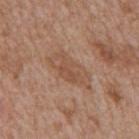Case summary:
– follow-up — total-body-photography surveillance lesion; no biopsy
– lesion diameter — ≈6 mm
– image-analysis metrics — a footprint of about 12 mm², an outline eccentricity of about 0.9 (0 = round, 1 = elongated), and a shape-asymmetry score of about 0.2 (0 = symmetric); an average lesion color of about L≈51 a*≈19 b*≈30 (CIELAB) and about 7 CIELAB-L* units darker than the surrounding skin; a border-irregularity rating of about 3/10; a lesion-detection confidence of about 100/100
– subject — male, approximately 65 years of age
– imaging modality — total-body-photography crop, ~15 mm field of view
– lighting — white-light
– site — the mid back